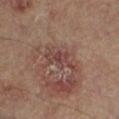follow-up=no biopsy performed (imaged during a skin exam)
subject=male, aged approximately 65
diameter=≈4.5 mm
imaging modality=15 mm crop, total-body photography
tile lighting=cross-polarized illumination
body site=the left lower leg